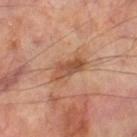Impression: The lesion was photographed on a routine skin check and not biopsied; there is no pathology result. Image and clinical context: The lesion is located on the left thigh. A 15 mm close-up extracted from a 3D total-body photography capture. An algorithmic analysis of the crop reported a mean CIELAB color near L≈50 a*≈23 b*≈32 and a normalized border contrast of about 7.5. The software also gave an automated nevus-likeness rating near 0 out of 100 and lesion-presence confidence of about 100/100. The lesion's longest dimension is about 4.5 mm. A male patient aged approximately 70. Imaged with cross-polarized lighting.